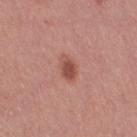Context:
The recorded lesion diameter is about 3 mm. A lesion tile, about 15 mm wide, cut from a 3D total-body photograph. The patient is a female aged 28 to 32. The tile uses white-light illumination. On the right thigh.